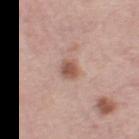<tbp_lesion>
<biopsy_status>not biopsied; imaged during a skin examination</biopsy_status>
<lesion_size>
  <long_diameter_mm_approx>2.5</long_diameter_mm_approx>
</lesion_size>
<lighting>white-light</lighting>
<image>
  <source>total-body photography crop</source>
  <field_of_view_mm>15</field_of_view_mm>
</image>
<site>left thigh</site>
<patient>
  <sex>female</sex>
  <age_approx>65</age_approx>
</patient>
</tbp_lesion>